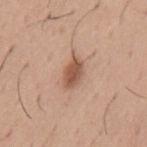biopsy status = no biopsy performed (imaged during a skin exam) | patient = male, aged 63–67 | anatomic site = the mid back | tile lighting = white-light illumination | lesion size = ~3.5 mm (longest diameter) | image source = total-body-photography crop, ~15 mm field of view.